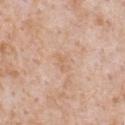lesion_size:
  long_diameter_mm_approx: 2.5
site: chest
image:
  source: total-body photography crop
  field_of_view_mm: 15
lighting: white-light
patient:
  sex: male
  age_approx: 65